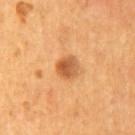Clinical impression:
The lesion was tiled from a total-body skin photograph and was not biopsied.